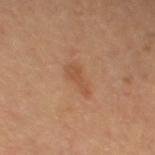Assessment:
This lesion was catalogued during total-body skin photography and was not selected for biopsy.
Clinical summary:
The total-body-photography lesion software estimated about 7 CIELAB-L* units darker than the surrounding skin and a lesion-to-skin contrast of about 5.5 (normalized; higher = more distinct). Longest diameter approximately 3 mm. The tile uses cross-polarized illumination. From the leg. This image is a 15 mm lesion crop taken from a total-body photograph. The subject is a female roughly 60 years of age.